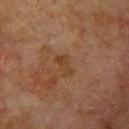| feature | finding |
|---|---|
| biopsy status | imaged on a skin check; not biopsied |
| size | ~3 mm (longest diameter) |
| site | the left upper arm |
| acquisition | ~15 mm crop, total-body skin-cancer survey |
| tile lighting | cross-polarized |
| automated metrics | a footprint of about 4 mm², an eccentricity of roughly 0.85, and a shape-asymmetry score of about 0.25 (0 = symmetric) |
| patient | male, approximately 75 years of age |